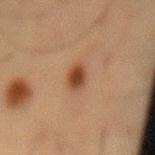Findings:
- notes: total-body-photography surveillance lesion; no biopsy
- automated metrics: a footprint of about 4.5 mm² and a symmetry-axis asymmetry near 0.25; border irregularity of about 2.5 on a 0–10 scale and a color-variation rating of about 3/10
- lighting: cross-polarized
- lesion diameter: about 3 mm
- image source: ~15 mm crop, total-body skin-cancer survey
- anatomic site: the mid back
- patient: male, about 60 years old